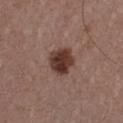Image and clinical context: A 15 mm close-up extracted from a 3D total-body photography capture. A male subject aged 38 to 42. From the chest. Captured under white-light illumination.